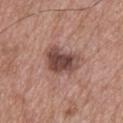{
  "biopsy_status": "not biopsied; imaged during a skin examination",
  "lesion_size": {
    "long_diameter_mm_approx": 4.5
  },
  "automated_metrics": {
    "area_mm2_approx": 12.0,
    "eccentricity": 0.75,
    "shape_asymmetry": 0.35,
    "vs_skin_darker_L": 14.0,
    "vs_skin_contrast_norm": 10.0,
    "border_irregularity_0_10": 3.5,
    "color_variation_0_10": 4.5,
    "peripheral_color_asymmetry": 1.5,
    "nevus_likeness_0_100": 30
  },
  "image": {
    "source": "total-body photography crop",
    "field_of_view_mm": 15
  },
  "lighting": "white-light",
  "patient": {
    "sex": "male",
    "age_approx": 65
  },
  "site": "upper back"
}